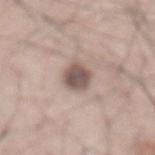Q: Automated lesion metrics?
A: a footprint of about 7 mm², an eccentricity of roughly 0.75, and a symmetry-axis asymmetry near 0.2; border irregularity of about 2 on a 0–10 scale, internal color variation of about 3.5 on a 0–10 scale, and peripheral color asymmetry of about 1
Q: Lesion location?
A: the abdomen
Q: Who is the patient?
A: male, aged around 65
Q: How large is the lesion?
A: ≈3.5 mm
Q: What lighting was used for the tile?
A: white-light illumination
Q: What kind of image is this?
A: ~15 mm crop, total-body skin-cancer survey